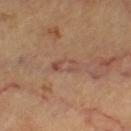Imaged during a routine full-body skin examination; the lesion was not biopsied and no histopathology is available.
This is a cross-polarized tile.
A female subject aged 58 to 62.
A 15 mm close-up tile from a total-body photography series done for melanoma screening.
From the leg.
The lesion-visualizer software estimated a mean CIELAB color near L≈50 a*≈21 b*≈27, roughly 6 lightness units darker than nearby skin, and a normalized border contrast of about 5.5. The analysis additionally found border irregularity of about 3 on a 0–10 scale and radial color variation of about 2. It also reported a nevus-likeness score of about 0/100 and a detector confidence of about 85 out of 100 that the crop contains a lesion.
The recorded lesion diameter is about 3.5 mm.